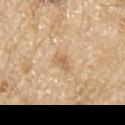Assessment: No biopsy was performed on this lesion — it was imaged during a full skin examination and was not determined to be concerning. Acquisition and patient details: A 15 mm crop from a total-body photograph taken for skin-cancer surveillance. Captured under white-light illumination. The total-body-photography lesion software estimated internal color variation of about 2.5 on a 0–10 scale and radial color variation of about 1. On the right upper arm. A male patient, roughly 70 years of age. Approximately 2.5 mm at its widest.